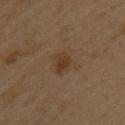notes — imaged on a skin check; not biopsied | patient — female, about 45 years old | tile lighting — cross-polarized | imaging modality — ~15 mm tile from a whole-body skin photo | body site — the back.